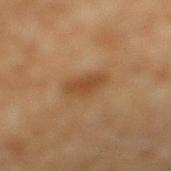Q: Was a biopsy performed?
A: catalogued during a skin exam; not biopsied
Q: How large is the lesion?
A: ~4 mm (longest diameter)
Q: Lesion location?
A: the right lower leg
Q: What kind of image is this?
A: ~15 mm crop, total-body skin-cancer survey
Q: What are the patient's age and sex?
A: male, approximately 60 years of age
Q: Illumination type?
A: cross-polarized
Q: What did automated image analysis measure?
A: an area of roughly 7 mm² and an outline eccentricity of about 0.8 (0 = round, 1 = elongated); a mean CIELAB color near L≈44 a*≈19 b*≈34; internal color variation of about 2 on a 0–10 scale and peripheral color asymmetry of about 0.5